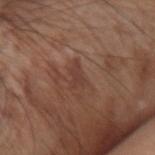Findings:
– follow-up: imaged on a skin check; not biopsied
– size: ≈2.5 mm
– image: 15 mm crop, total-body photography
– anatomic site: the left forearm
– subject: male, aged 68–72
– image-analysis metrics: a mean CIELAB color near L≈39 a*≈20 b*≈24, a lesion–skin lightness drop of about 7, and a normalized lesion–skin contrast near 6; internal color variation of about 0 on a 0–10 scale and radial color variation of about 0; a classifier nevus-likeness of about 0/100